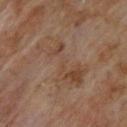Assessment:
The lesion was tiled from a total-body skin photograph and was not biopsied.
Acquisition and patient details:
The patient is a male aged around 70. The tile uses cross-polarized illumination. The recorded lesion diameter is about 8 mm. Located on the back. A lesion tile, about 15 mm wide, cut from a 3D total-body photograph.